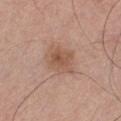Q: Was a biopsy performed?
A: total-body-photography surveillance lesion; no biopsy
Q: Illumination type?
A: white-light
Q: What are the patient's age and sex?
A: male, aged approximately 30
Q: How large is the lesion?
A: about 4 mm
Q: Lesion location?
A: the chest
Q: What kind of image is this?
A: ~15 mm crop, total-body skin-cancer survey